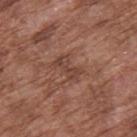Q: Is there a histopathology result?
A: no biopsy performed (imaged during a skin exam)
Q: How was this image acquired?
A: ~15 mm crop, total-body skin-cancer survey
Q: Lesion location?
A: the upper back
Q: How large is the lesion?
A: about 3.5 mm
Q: What are the patient's age and sex?
A: male, in their mid-70s
Q: What did automated image analysis measure?
A: a lesion area of about 4.5 mm², an outline eccentricity of about 0.9 (0 = round, 1 = elongated), and a symmetry-axis asymmetry near 0.35; a border-irregularity index near 5/10, internal color variation of about 3 on a 0–10 scale, and radial color variation of about 1; an automated nevus-likeness rating near 0 out of 100
Q: Illumination type?
A: white-light illumination